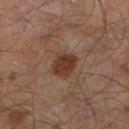{"biopsy_status": "not biopsied; imaged during a skin examination", "lighting": "cross-polarized", "image": {"source": "total-body photography crop", "field_of_view_mm": 15}, "site": "left lower leg", "patient": {"sex": "male", "age_approx": 65}}